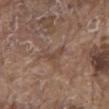| field | value |
|---|---|
| follow-up | imaged on a skin check; not biopsied |
| location | the mid back |
| patient | male, approximately 80 years of age |
| lighting | white-light |
| acquisition | total-body-photography crop, ~15 mm field of view |